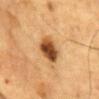follow-up — total-body-photography surveillance lesion; no biopsy | body site — the front of the torso | patient — male, in their mid- to late 80s or older | lesion diameter — about 4 mm | acquisition — total-body-photography crop, ~15 mm field of view | tile lighting — cross-polarized illumination.